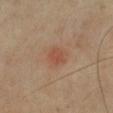Impression:
Imaged during a routine full-body skin examination; the lesion was not biopsied and no histopathology is available.